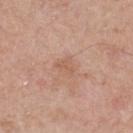Recorded during total-body skin imaging; not selected for excision or biopsy. Automated tile analysis of the lesion measured border irregularity of about 4.5 on a 0–10 scale, a color-variation rating of about 1/10, and a peripheral color-asymmetry measure near 0.5. The software also gave a classifier nevus-likeness of about 0/100 and lesion-presence confidence of about 100/100. The patient is a male roughly 55 years of age. This image is a 15 mm lesion crop taken from a total-body photograph. Located on the upper back. Measured at roughly 2.5 mm in maximum diameter. This is a white-light tile.